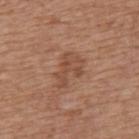biopsy status: total-body-photography surveillance lesion; no biopsy
lesion diameter: ~4.5 mm (longest diameter)
illumination: white-light illumination
subject: male, roughly 65 years of age
image-analysis metrics: an average lesion color of about L≈49 a*≈21 b*≈30 (CIELAB), roughly 7 lightness units darker than nearby skin, and a normalized border contrast of about 5.5; a nevus-likeness score of about 5/100
imaging modality: ~15 mm tile from a whole-body skin photo
location: the mid back